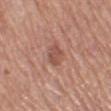| feature | finding |
|---|---|
| subject | female, in their mid-50s |
| location | the left thigh |
| imaging modality | ~15 mm tile from a whole-body skin photo |
| diameter | about 3 mm |